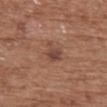Captured during whole-body skin photography for melanoma surveillance; the lesion was not biopsied.
Automated image analysis of the tile measured a footprint of about 4.5 mm² and a shape-asymmetry score of about 0.35 (0 = symmetric). The software also gave a lesion color around L≈44 a*≈20 b*≈26 in CIELAB, roughly 9 lightness units darker than nearby skin, and a lesion-to-skin contrast of about 7 (normalized; higher = more distinct). The software also gave a border-irregularity index near 4/10, internal color variation of about 2.5 on a 0–10 scale, and a peripheral color-asymmetry measure near 0.5.
The lesion is located on the back.
The recorded lesion diameter is about 3.5 mm.
A female patient, approximately 75 years of age.
This image is a 15 mm lesion crop taken from a total-body photograph.